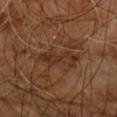Recorded during total-body skin imaging; not selected for excision or biopsy.
The patient is a male aged 58–62.
Imaged with cross-polarized lighting.
From the right arm.
Approximately 5 mm at its widest.
A close-up tile cropped from a whole-body skin photograph, about 15 mm across.